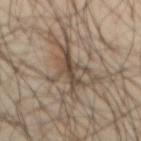<case>
<biopsy_status>not biopsied; imaged during a skin examination</biopsy_status>
<patient>
  <sex>male</sex>
  <age_approx>40</age_approx>
</patient>
<image>
  <source>total-body photography crop</source>
  <field_of_view_mm>15</field_of_view_mm>
</image>
<site>mid back</site>
<lighting>cross-polarized</lighting>
<lesion_size>
  <long_diameter_mm_approx>5.0</long_diameter_mm_approx>
</lesion_size>
<automated_metrics>
  <eccentricity>0.9</eccentricity>
  <shape_asymmetry>0.5</shape_asymmetry>
  <cielab_L>45</cielab_L>
  <cielab_a>12</cielab_a>
  <cielab_b>25</cielab_b>
  <vs_skin_darker_L>11.0</vs_skin_darker_L>
  <vs_skin_contrast_norm>8.5</vs_skin_contrast_norm>
  <border_irregularity_0_10>7.5</border_irregularity_0_10>
  <color_variation_0_10>2.5</color_variation_0_10>
  <peripheral_color_asymmetry>1.0</peripheral_color_asymmetry>
  <nevus_likeness_0_100>0</nevus_likeness_0_100>
</automated_metrics>
</case>